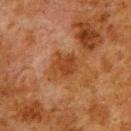Q: Is there a histopathology result?
A: no biopsy performed (imaged during a skin exam)